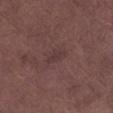This lesion was catalogued during total-body skin photography and was not selected for biopsy.
The lesion is located on the left lower leg.
A 15 mm close-up extracted from a 3D total-body photography capture.
A male subject in their mid- to late 50s.
This is a white-light tile.